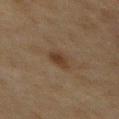{
  "patient": {
    "sex": "male",
    "age_approx": 75
  },
  "lesion_size": {
    "long_diameter_mm_approx": 2.5
  },
  "image": {
    "source": "total-body photography crop",
    "field_of_view_mm": 15
  },
  "site": "front of the torso",
  "automated_metrics": {
    "color_variation_0_10": 2.0,
    "lesion_detection_confidence_0_100": 100
  }
}